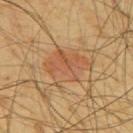{
  "biopsy_status": "not biopsied; imaged during a skin examination",
  "site": "back",
  "image": {
    "source": "total-body photography crop",
    "field_of_view_mm": 15
  },
  "lighting": "cross-polarized",
  "automated_metrics": {
    "border_irregularity_0_10": 3.5,
    "color_variation_0_10": 4.5,
    "peripheral_color_asymmetry": 1.5
  },
  "patient": {
    "sex": "male",
    "age_approx": 65
  }
}